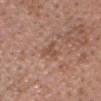Recorded during total-body skin imaging; not selected for excision or biopsy. Cropped from a total-body skin-imaging series; the visible field is about 15 mm. The patient is a male about 55 years old. The tile uses white-light illumination. The lesion is on the head or neck.